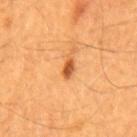Impression: Part of a total-body skin-imaging series; this lesion was reviewed on a skin check and was not flagged for biopsy. Image and clinical context: An algorithmic analysis of the crop reported an average lesion color of about L≈53 a*≈27 b*≈42 (CIELAB). The analysis additionally found peripheral color asymmetry of about 0.5. Approximately 2.5 mm at its widest. The tile uses cross-polarized illumination. From the mid back. A 15 mm crop from a total-body photograph taken for skin-cancer surveillance. A male subject aged 58–62.